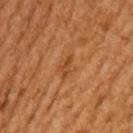Q: Was a biopsy performed?
A: no biopsy performed (imaged during a skin exam)
Q: What kind of image is this?
A: 15 mm crop, total-body photography
Q: Lesion location?
A: the left upper arm
Q: Patient demographics?
A: female, in their mid-50s
Q: Illumination type?
A: cross-polarized